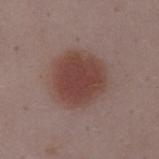The lesion was photographed on a routine skin check and not biopsied; there is no pathology result.
Automated image analysis of the tile measured an eccentricity of roughly 0.3. And it measured an average lesion color of about L≈42 a*≈20 b*≈22 (CIELAB). It also reported a border-irregularity index near 1.5/10, a within-lesion color-variation index near 3.5/10, and radial color variation of about 1.
A lesion tile, about 15 mm wide, cut from a 3D total-body photograph.
Measured at roughly 6 mm in maximum diameter.
This is a white-light tile.
On the abdomen.
The patient is a female aged 33 to 37.